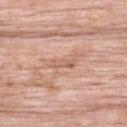The lesion was photographed on a routine skin check and not biopsied; there is no pathology result. The subject is a female in their 70s. The lesion is located on the back. An algorithmic analysis of the crop reported an automated nevus-likeness rating near 0 out of 100. A roughly 15 mm field-of-view crop from a total-body skin photograph. Imaged with white-light lighting.